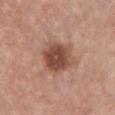Assessment:
The lesion was photographed on a routine skin check and not biopsied; there is no pathology result.
Acquisition and patient details:
This is a white-light tile. Measured at roughly 5 mm in maximum diameter. A 15 mm crop from a total-body photograph taken for skin-cancer surveillance. A female patient approximately 55 years of age. The lesion is on the chest.